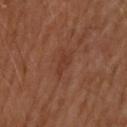The tile uses cross-polarized illumination.
The lesion-visualizer software estimated a lesion area of about 3.5 mm², an outline eccentricity of about 0.85 (0 = round, 1 = elongated), and a symmetry-axis asymmetry near 0.35. The software also gave a lesion color around L≈34 a*≈23 b*≈27 in CIELAB and a normalized lesion–skin contrast near 5. The software also gave a border-irregularity rating of about 3.5/10, internal color variation of about 2 on a 0–10 scale, and peripheral color asymmetry of about 1.
The lesion is located on the upper back.
A 15 mm crop from a total-body photograph taken for skin-cancer surveillance.
The recorded lesion diameter is about 3 mm.
A male patient, aged 63–67.